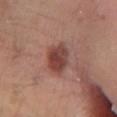Impression:
Imaged during a routine full-body skin examination; the lesion was not biopsied and no histopathology is available.
Context:
A 15 mm close-up tile from a total-body photography series done for melanoma screening. A male patient, in their mid- to late 60s. Located on the left lower leg. About 4 mm across.